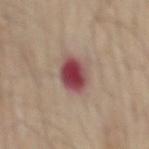This lesion was catalogued during total-body skin photography and was not selected for biopsy. A male subject, in their 70s. A 15 mm crop from a total-body photograph taken for skin-cancer surveillance. Measured at roughly 3.5 mm in maximum diameter. This is a white-light tile. The lesion is on the mid back. Automated tile analysis of the lesion measured a lesion area of about 9.5 mm², a shape eccentricity near 0.4, and a shape-asymmetry score of about 0.1 (0 = symmetric). The software also gave an average lesion color of about L≈46 a*≈28 b*≈20 (CIELAB).